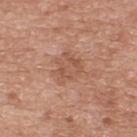Case summary:
• biopsy status · no biopsy performed (imaged during a skin exam)
• imaging modality · total-body-photography crop, ~15 mm field of view
• patient · male, approximately 50 years of age
• diameter · about 4 mm
• illumination · white-light
• body site · the upper back